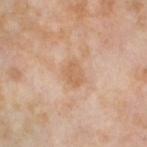| field | value |
|---|---|
| notes | total-body-photography surveillance lesion; no biopsy |
| tile lighting | cross-polarized illumination |
| patient | female, in their mid-50s |
| size | about 3 mm |
| image | 15 mm crop, total-body photography |
| anatomic site | the leg |